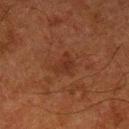Part of a total-body skin-imaging series; this lesion was reviewed on a skin check and was not flagged for biopsy. Located on the left lower leg. The tile uses cross-polarized illumination. This image is a 15 mm lesion crop taken from a total-body photograph. The lesion-visualizer software estimated a mean CIELAB color near L≈25 a*≈19 b*≈25 and a normalized border contrast of about 5. The lesion's longest dimension is about 3 mm. The patient is a male aged approximately 80.